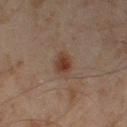site: leg
image:
  source: total-body photography crop
  field_of_view_mm: 15
automated_metrics:
  area_mm2_approx: 4.0
  eccentricity: 0.7
  shape_asymmetry: 0.15
patient:
  sex: male
  age_approx: 45
lesion_size:
  long_diameter_mm_approx: 2.5
lighting: cross-polarized